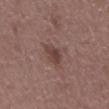The lesion was tiled from a total-body skin photograph and was not biopsied.
A male subject, in their 60s.
The tile uses white-light illumination.
A lesion tile, about 15 mm wide, cut from a 3D total-body photograph.
Measured at roughly 3 mm in maximum diameter.
From the chest.
The total-body-photography lesion software estimated an area of roughly 4.5 mm² and two-axis asymmetry of about 0.25. And it measured a mean CIELAB color near L≈41 a*≈19 b*≈21, about 9 CIELAB-L* units darker than the surrounding skin, and a normalized lesion–skin contrast near 7.5. And it measured an automated nevus-likeness rating near 30 out of 100 and a lesion-detection confidence of about 100/100.